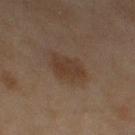Recorded during total-body skin imaging; not selected for excision or biopsy. Located on the left thigh. The total-body-photography lesion software estimated about 6 CIELAB-L* units darker than the surrounding skin. The lesion's longest dimension is about 4 mm. Cropped from a whole-body photographic skin survey; the tile spans about 15 mm. A female subject, approximately 60 years of age.